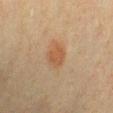follow-up: no biopsy performed (imaged during a skin exam) | subject: male, aged 38–42 | lighting: cross-polarized illumination | acquisition: total-body-photography crop, ~15 mm field of view | location: the chest | lesion diameter: ~2.5 mm (longest diameter).